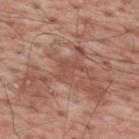Q: Is there a histopathology result?
A: no biopsy performed (imaged during a skin exam)
Q: What did automated image analysis measure?
A: an area of roughly 4 mm², an outline eccentricity of about 0.8 (0 = round, 1 = elongated), and a symmetry-axis asymmetry near 0.55; an average lesion color of about L≈50 a*≈22 b*≈29 (CIELAB) and a normalized border contrast of about 4.5; an automated nevus-likeness rating near 0 out of 100 and a detector confidence of about 55 out of 100 that the crop contains a lesion
Q: What is the anatomic site?
A: the upper back
Q: What is the lesion's diameter?
A: about 3.5 mm
Q: What kind of image is this?
A: 15 mm crop, total-body photography
Q: What lighting was used for the tile?
A: white-light illumination
Q: Patient demographics?
A: male, aged 68 to 72